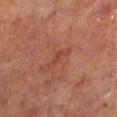Part of a total-body skin-imaging series; this lesion was reviewed on a skin check and was not flagged for biopsy.
A 15 mm crop from a total-body photograph taken for skin-cancer surveillance.
The lesion's longest dimension is about 3.5 mm.
A male patient in their 70s.
The lesion is located on the left lower leg.